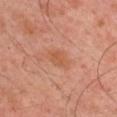Assessment:
No biopsy was performed on this lesion — it was imaged during a full skin examination and was not determined to be concerning.
Background:
A male subject, aged approximately 45. The lesion-visualizer software estimated an eccentricity of roughly 0.75 and a symmetry-axis asymmetry near 0.3. The software also gave about 6 CIELAB-L* units darker than the surrounding skin and a lesion-to-skin contrast of about 6 (normalized; higher = more distinct). The analysis additionally found a border-irregularity rating of about 2.5/10 and radial color variation of about 0.5. It also reported an automated nevus-likeness rating near 0 out of 100 and lesion-presence confidence of about 100/100. From the arm. The tile uses cross-polarized illumination. Longest diameter approximately 2.5 mm. A 15 mm crop from a total-body photograph taken for skin-cancer surveillance.